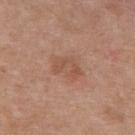Part of a total-body skin-imaging series; this lesion was reviewed on a skin check and was not flagged for biopsy.
Located on the upper back.
A region of skin cropped from a whole-body photographic capture, roughly 15 mm wide.
An algorithmic analysis of the crop reported a within-lesion color-variation index near 2.5/10 and a peripheral color-asymmetry measure near 1. It also reported a nevus-likeness score of about 10/100 and a detector confidence of about 100 out of 100 that the crop contains a lesion.
A female patient approximately 40 years of age.
This is a white-light tile.
Approximately 3.5 mm at its widest.